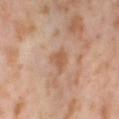The lesion was photographed on a routine skin check and not biopsied; there is no pathology result. A female patient aged 53–57. A roughly 15 mm field-of-view crop from a total-body skin photograph. The lesion-visualizer software estimated an average lesion color of about L≈57 a*≈21 b*≈33 (CIELAB) and roughly 8 lightness units darker than nearby skin. Approximately 2.5 mm at its widest. The lesion is located on the left thigh.